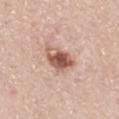| field | value |
|---|---|
| biopsy status | total-body-photography surveillance lesion; no biopsy |
| automated lesion analysis | a symmetry-axis asymmetry near 0.25; a border-irregularity rating of about 2.5/10, internal color variation of about 8 on a 0–10 scale, and a peripheral color-asymmetry measure near 3 |
| imaging modality | ~15 mm crop, total-body skin-cancer survey |
| lighting | white-light illumination |
| location | the mid back |
| subject | male, aged approximately 40 |
| size | ~4 mm (longest diameter) |